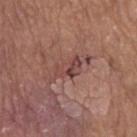Imaged during a routine full-body skin examination; the lesion was not biopsied and no histopathology is available. The patient is a male aged approximately 80. This is a white-light tile. Longest diameter approximately 3.5 mm. Located on the arm. The lesion-visualizer software estimated a lesion area of about 5 mm², an outline eccentricity of about 0.85 (0 = round, 1 = elongated), and a symmetry-axis asymmetry near 0.4. It also reported about 8 CIELAB-L* units darker than the surrounding skin and a normalized lesion–skin contrast near 7. And it measured an automated nevus-likeness rating near 0 out of 100 and a lesion-detection confidence of about 80/100. A lesion tile, about 15 mm wide, cut from a 3D total-body photograph.